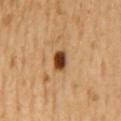The lesion was photographed on a routine skin check and not biopsied; there is no pathology result. The lesion is located on the back. Captured under cross-polarized illumination. A female subject, in their mid-50s. Automated image analysis of the tile measured a footprint of about 4.5 mm², a shape eccentricity near 0.8, and two-axis asymmetry of about 0.2. It also reported a lesion color around L≈45 a*≈23 b*≈37 in CIELAB and a lesion-to-skin contrast of about 14 (normalized; higher = more distinct). The analysis additionally found a border-irregularity index near 2/10 and a within-lesion color-variation index near 4.5/10. A region of skin cropped from a whole-body photographic capture, roughly 15 mm wide. Measured at roughly 3 mm in maximum diameter.